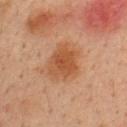No biopsy was performed on this lesion — it was imaged during a full skin examination and was not determined to be concerning. From the back. Captured under cross-polarized illumination. A 15 mm crop from a total-body photograph taken for skin-cancer surveillance. The lesion's longest dimension is about 4.5 mm. Automated image analysis of the tile measured a lesion area of about 13 mm², an eccentricity of roughly 0.5, and two-axis asymmetry of about 0.2. It also reported an average lesion color of about L≈48 a*≈22 b*≈34 (CIELAB) and a lesion-to-skin contrast of about 7.5 (normalized; higher = more distinct). The software also gave a border-irregularity index near 2.5/10, a within-lesion color-variation index near 3.5/10, and a peripheral color-asymmetry measure near 1. The software also gave a nevus-likeness score of about 70/100 and lesion-presence confidence of about 100/100. The patient is a male aged 33–37.